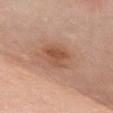* follow-up · total-body-photography surveillance lesion; no biopsy
* anatomic site · the chest
* acquisition · 15 mm crop, total-body photography
* automated lesion analysis · an outline eccentricity of about 0.6 (0 = round, 1 = elongated) and two-axis asymmetry of about 0.3; a border-irregularity rating of about 3/10; a nevus-likeness score of about 25/100 and a lesion-detection confidence of about 100/100
* lighting · white-light illumination
* patient · female, aged around 50
* diameter · ≈3.5 mm